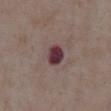| feature | finding |
|---|---|
| biopsy status | no biopsy performed (imaged during a skin exam) |
| body site | the abdomen |
| tile lighting | white-light illumination |
| image source | ~15 mm tile from a whole-body skin photo |
| subject | male, in their mid- to late 70s |
| lesion diameter | about 2.5 mm |
| automated lesion analysis | border irregularity of about 1 on a 0–10 scale and a within-lesion color-variation index near 3.5/10; a nevus-likeness score of about 0/100 and a lesion-detection confidence of about 100/100 |